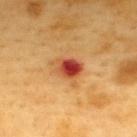Clinical impression: The lesion was tiled from a total-body skin photograph and was not biopsied. Background: The tile uses cross-polarized illumination. Longest diameter approximately 3.5 mm. The subject is a male about 60 years old. An algorithmic analysis of the crop reported an outline eccentricity of about 0.7 (0 = round, 1 = elongated) and a symmetry-axis asymmetry near 0.25. And it measured a mean CIELAB color near L≈50 a*≈33 b*≈40, roughly 16 lightness units darker than nearby skin, and a normalized border contrast of about 11. The software also gave a classifier nevus-likeness of about 0/100 and a detector confidence of about 100 out of 100 that the crop contains a lesion. The lesion is on the upper back. A 15 mm close-up extracted from a 3D total-body photography capture.